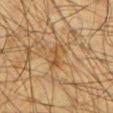Notes:
- workup · total-body-photography surveillance lesion; no biopsy
- site · the left lower leg
- lesion diameter · about 2.5 mm
- imaging modality · ~15 mm crop, total-body skin-cancer survey
- patient · male, in their mid- to late 30s
- TBP lesion metrics · a footprint of about 3.5 mm², an outline eccentricity of about 0.85 (0 = round, 1 = elongated), and a symmetry-axis asymmetry near 0.5; internal color variation of about 1 on a 0–10 scale and peripheral color asymmetry of about 0; an automated nevus-likeness rating near 0 out of 100 and lesion-presence confidence of about 90/100
- tile lighting · cross-polarized illumination